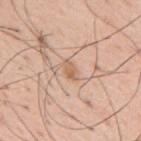biopsy status — catalogued during a skin exam; not biopsied | tile lighting — white-light illumination | image — ~15 mm crop, total-body skin-cancer survey | subject — male, about 55 years old | automated lesion analysis — a lesion color around L≈63 a*≈19 b*≈33 in CIELAB, about 8 CIELAB-L* units darker than the surrounding skin, and a lesion-to-skin contrast of about 6 (normalized; higher = more distinct); a border-irregularity rating of about 2/10, a color-variation rating of about 3/10, and peripheral color asymmetry of about 1 | anatomic site — the mid back | lesion size — ~2.5 mm (longest diameter).